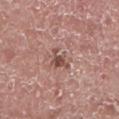Clinical impression: This lesion was catalogued during total-body skin photography and was not selected for biopsy. Clinical summary: A male subject roughly 75 years of age. Cropped from a total-body skin-imaging series; the visible field is about 15 mm. The recorded lesion diameter is about 3 mm. Imaged with white-light lighting. Located on the left lower leg.